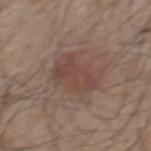| feature | finding |
|---|---|
| follow-up | total-body-photography surveillance lesion; no biopsy |
| image source | total-body-photography crop, ~15 mm field of view |
| patient | male, in their 50s |
| site | the mid back |